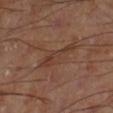follow-up: no biopsy performed (imaged during a skin exam)
location: the leg
acquisition: ~15 mm tile from a whole-body skin photo